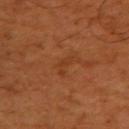{"biopsy_status": "not biopsied; imaged during a skin examination", "image": {"source": "total-body photography crop", "field_of_view_mm": 15}, "patient": {"sex": "male", "age_approx": 50}, "site": "right upper arm"}